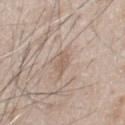Q: Was this lesion biopsied?
A: catalogued during a skin exam; not biopsied
Q: What is the lesion's diameter?
A: about 3 mm
Q: How was this image acquired?
A: ~15 mm crop, total-body skin-cancer survey
Q: How was the tile lit?
A: white-light
Q: What are the patient's age and sex?
A: male, approximately 50 years of age
Q: What is the anatomic site?
A: the chest